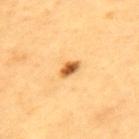notes: no biopsy performed (imaged during a skin exam)
anatomic site: the upper back
tile lighting: cross-polarized
diameter: ≈2.5 mm
patient: male, roughly 60 years of age
image source: ~15 mm crop, total-body skin-cancer survey
image-analysis metrics: about 18 CIELAB-L* units darker than the surrounding skin and a lesion-to-skin contrast of about 10.5 (normalized; higher = more distinct); a border-irregularity rating of about 2/10 and a color-variation rating of about 5/10; a nevus-likeness score of about 100/100 and lesion-presence confidence of about 100/100